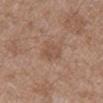The total-body-photography lesion software estimated a footprint of about 5 mm² and an eccentricity of roughly 0.7. And it measured an average lesion color of about L≈51 a*≈19 b*≈27 (CIELAB) and a normalized border contrast of about 4.5. Cropped from a whole-body photographic skin survey; the tile spans about 15 mm. The lesion is located on the mid back. The subject is a male about 75 years old.